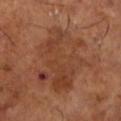Findings:
• follow-up · no biopsy performed (imaged during a skin exam)
• illumination · cross-polarized illumination
• lesion diameter · about 7.5 mm
• acquisition · 15 mm crop, total-body photography
• subject · male, about 65 years old
• automated lesion analysis · a border-irregularity rating of about 5.5/10 and internal color variation of about 5.5 on a 0–10 scale
• location · the left lower leg